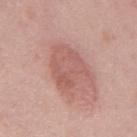| key | value |
|---|---|
| notes | no biopsy performed (imaged during a skin exam) |
| anatomic site | the back |
| size | ~5 mm (longest diameter) |
| image | 15 mm crop, total-body photography |
| subject | male, aged 43–47 |
| lighting | white-light illumination |
| image-analysis metrics | a lesion color around L≈58 a*≈24 b*≈25 in CIELAB, roughly 8 lightness units darker than nearby skin, and a normalized lesion–skin contrast near 5.5; a classifier nevus-likeness of about 45/100 and a detector confidence of about 100 out of 100 that the crop contains a lesion |